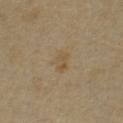{"biopsy_status": "not biopsied; imaged during a skin examination", "image": {"source": "total-body photography crop", "field_of_view_mm": 15}, "lesion_size": {"long_diameter_mm_approx": 3.5}, "patient": {"sex": "female", "age_approx": 50}, "site": "chest"}